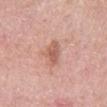Assessment: The lesion was photographed on a routine skin check and not biopsied; there is no pathology result. Image and clinical context: A female subject about 60 years old. The lesion is on the mid back. A roughly 15 mm field-of-view crop from a total-body skin photograph.